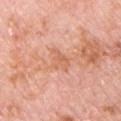body site: the front of the torso | subject: male, aged around 80 | size: ~3 mm (longest diameter) | illumination: white-light | image: ~15 mm crop, total-body skin-cancer survey.